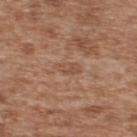Assessment:
Imaged during a routine full-body skin examination; the lesion was not biopsied and no histopathology is available.
Acquisition and patient details:
A close-up tile cropped from a whole-body skin photograph, about 15 mm across. The recorded lesion diameter is about 2.5 mm. The subject is a male aged 63–67. On the upper back.